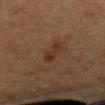Captured during whole-body skin photography for melanoma surveillance; the lesion was not biopsied.
The lesion-visualizer software estimated a footprint of about 4 mm², an outline eccentricity of about 0.8 (0 = round, 1 = elongated), and two-axis asymmetry of about 0.3. The software also gave a border-irregularity rating of about 3/10 and peripheral color asymmetry of about 1. And it measured a classifier nevus-likeness of about 80/100 and a detector confidence of about 100 out of 100 that the crop contains a lesion.
About 3 mm across.
On the back.
Captured under cross-polarized illumination.
A female subject, aged 38 to 42.
A 15 mm close-up tile from a total-body photography series done for melanoma screening.